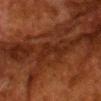Captured during whole-body skin photography for melanoma surveillance; the lesion was not biopsied.
An algorithmic analysis of the crop reported a lesion color around L≈20 a*≈20 b*≈24 in CIELAB and a normalized border contrast of about 5. The software also gave border irregularity of about 7 on a 0–10 scale and a within-lesion color-variation index near 0.5/10. The software also gave a nevus-likeness score of about 0/100 and a detector confidence of about 75 out of 100 that the crop contains a lesion.
The lesion is on the head or neck.
The tile uses cross-polarized illumination.
Measured at roughly 3 mm in maximum diameter.
A male subject, approximately 80 years of age.
This image is a 15 mm lesion crop taken from a total-body photograph.